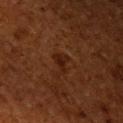No biopsy was performed on this lesion — it was imaged during a full skin examination and was not determined to be concerning. A male patient, roughly 60 years of age. The recorded lesion diameter is about 2.5 mm. This image is a 15 mm lesion crop taken from a total-body photograph. The lesion is on the left upper arm. The lesion-visualizer software estimated a footprint of about 3 mm², an eccentricity of roughly 0.75, and two-axis asymmetry of about 0.55. The software also gave an automated nevus-likeness rating near 80 out of 100 and a lesion-detection confidence of about 100/100. This is a cross-polarized tile.